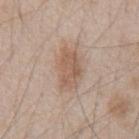Q: Was a biopsy performed?
A: catalogued during a skin exam; not biopsied
Q: What is the lesion's diameter?
A: about 4.5 mm
Q: Automated lesion metrics?
A: a border-irregularity index near 2.5/10, a within-lesion color-variation index near 3/10, and peripheral color asymmetry of about 1; a lesion-detection confidence of about 100/100
Q: Lesion location?
A: the front of the torso
Q: What are the patient's age and sex?
A: male, in their mid- to late 40s
Q: What lighting was used for the tile?
A: white-light
Q: What kind of image is this?
A: ~15 mm crop, total-body skin-cancer survey